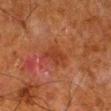Impression: No biopsy was performed on this lesion — it was imaged during a full skin examination and was not determined to be concerning. Acquisition and patient details: A male patient, aged approximately 80. The recorded lesion diameter is about 3 mm. A lesion tile, about 15 mm wide, cut from a 3D total-body photograph. An algorithmic analysis of the crop reported a shape eccentricity near 0.75 and a shape-asymmetry score of about 0.3 (0 = symmetric). And it measured border irregularity of about 3.5 on a 0–10 scale and a within-lesion color-variation index near 1/10. On the right lower leg.